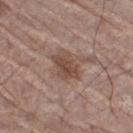The lesion was photographed on a routine skin check and not biopsied; there is no pathology result.
The patient is a male in their mid- to late 60s.
About 4 mm across.
On the right thigh.
Automated image analysis of the tile measured about 9 CIELAB-L* units darker than the surrounding skin and a normalized border contrast of about 7.5.
Imaged with white-light lighting.
A region of skin cropped from a whole-body photographic capture, roughly 15 mm wide.